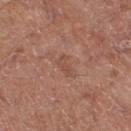workup: no biopsy performed (imaged during a skin exam) | image source: 15 mm crop, total-body photography | tile lighting: white-light | subject: male, aged 63 to 67 | body site: the right thigh | TBP lesion metrics: a lesion area of about 4 mm²; a lesion–skin lightness drop of about 6 and a lesion-to-skin contrast of about 4.5 (normalized; higher = more distinct); a border-irregularity index near 3.5/10, a color-variation rating of about 1.5/10, and peripheral color asymmetry of about 0.5; a lesion-detection confidence of about 100/100 | diameter: ≈3 mm.